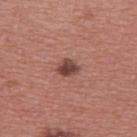The lesion was photographed on a routine skin check and not biopsied; there is no pathology result. A region of skin cropped from a whole-body photographic capture, roughly 15 mm wide. The lesion's longest dimension is about 2.5 mm. A male patient, aged 38 to 42. On the upper back.